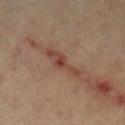Recorded during total-body skin imaging; not selected for excision or biopsy. A close-up tile cropped from a whole-body skin photograph, about 15 mm across. The total-body-photography lesion software estimated an average lesion color of about L≈44 a*≈21 b*≈26 (CIELAB) and a normalized lesion–skin contrast near 7. It also reported a border-irregularity rating of about 5.5/10, a within-lesion color-variation index near 3.5/10, and radial color variation of about 0.5. The subject is about 60 years old. About 5.5 mm across. Imaged with cross-polarized lighting. The lesion is located on the left lower leg.